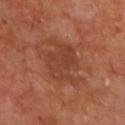Assessment:
Captured during whole-body skin photography for melanoma surveillance; the lesion was not biopsied.
Background:
The lesion is located on the chest. Automated tile analysis of the lesion measured an area of roughly 16 mm², an eccentricity of roughly 0.65, and two-axis asymmetry of about 0.35. The subject is a male aged around 65. Measured at roughly 6 mm in maximum diameter. Cropped from a total-body skin-imaging series; the visible field is about 15 mm. This is a cross-polarized tile.